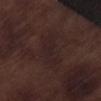Notes:
• follow-up: catalogued during a skin exam; not biopsied
• tile lighting: white-light
• location: the left lower leg
• imaging modality: total-body-photography crop, ~15 mm field of view
• diameter: ~5 mm (longest diameter)
• subject: male, aged around 70
• TBP lesion metrics: a lesion area of about 9.5 mm²; a lesion color around L≈21 a*≈15 b*≈14 in CIELAB; border irregularity of about 4 on a 0–10 scale, internal color variation of about 2 on a 0–10 scale, and a peripheral color-asymmetry measure near 0.5